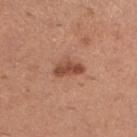notes=imaged on a skin check; not biopsied | patient=female, aged around 30 | anatomic site=the left lower leg | diameter=≈3.5 mm | image=15 mm crop, total-body photography | lighting=white-light illumination | image-analysis metrics=a footprint of about 5.5 mm², a shape eccentricity near 0.85, and a symmetry-axis asymmetry near 0.3; an average lesion color of about L≈49 a*≈25 b*≈31 (CIELAB) and roughly 12 lightness units darker than nearby skin; a border-irregularity rating of about 3.5/10, a within-lesion color-variation index near 3/10, and radial color variation of about 1; lesion-presence confidence of about 100/100.